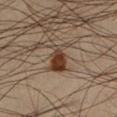{"patient": {"sex": "male", "age_approx": 35}, "lighting": "cross-polarized", "image": {"source": "total-body photography crop", "field_of_view_mm": 15}, "site": "leg", "lesion_size": {"long_diameter_mm_approx": 4.0}}